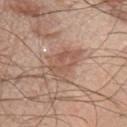– acquisition · total-body-photography crop, ~15 mm field of view
– body site · the upper back
– size · ~5 mm (longest diameter)
– patient · male, in their mid-60s
– tile lighting · white-light illumination
– automated metrics · an outline eccentricity of about 0.8 (0 = round, 1 = elongated) and a symmetry-axis asymmetry near 0.35